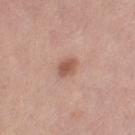Clinical impression: This lesion was catalogued during total-body skin photography and was not selected for biopsy. Acquisition and patient details: A close-up tile cropped from a whole-body skin photograph, about 15 mm across. Automated tile analysis of the lesion measured a shape eccentricity near 0.65 and two-axis asymmetry of about 0.15. And it measured an average lesion color of about L≈55 a*≈22 b*≈28 (CIELAB) and a normalized lesion–skin contrast near 7.5. The analysis additionally found a border-irregularity index near 1.5/10, internal color variation of about 2 on a 0–10 scale, and radial color variation of about 0.5. The tile uses white-light illumination. Measured at roughly 2.5 mm in maximum diameter. A female subject, aged approximately 35. From the left thigh.